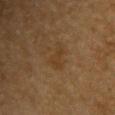notes: no biopsy performed (imaged during a skin exam)
lighting: cross-polarized
size: about 3 mm
site: the upper back
acquisition: ~15 mm crop, total-body skin-cancer survey
subject: male, aged 83 or over